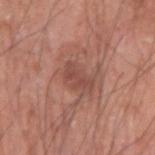Notes:
• workup · catalogued during a skin exam; not biopsied
• subject · male, aged 73 to 77
• lighting · white-light illumination
• body site · the right upper arm
• imaging modality · ~15 mm tile from a whole-body skin photo